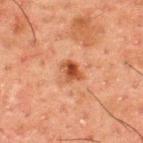No biopsy was performed on this lesion — it was imaged during a full skin examination and was not determined to be concerning.
A 15 mm close-up tile from a total-body photography series done for melanoma screening.
A male subject, about 50 years old.
The recorded lesion diameter is about 2.5 mm.
The lesion-visualizer software estimated an area of roughly 3.5 mm², an outline eccentricity of about 0.7 (0 = round, 1 = elongated), and a symmetry-axis asymmetry near 0.3. And it measured a lesion–skin lightness drop of about 11 and a normalized border contrast of about 9.5. It also reported internal color variation of about 3.5 on a 0–10 scale and a peripheral color-asymmetry measure near 1.5.
The lesion is located on the upper back.